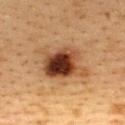{"biopsy_status": "not biopsied; imaged during a skin examination", "patient": {"sex": "female", "age_approx": 40}, "site": "upper back", "lesion_size": {"long_diameter_mm_approx": 5.5}, "automated_metrics": {"area_mm2_approx": 18.0, "shape_asymmetry": 0.3, "border_irregularity_0_10": 3.5, "color_variation_0_10": 10.0, "nevus_likeness_0_100": 100, "lesion_detection_confidence_0_100": 100}, "image": {"source": "total-body photography crop", "field_of_view_mm": 15}, "lighting": "cross-polarized"}